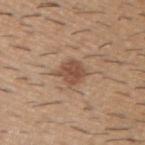This lesion was catalogued during total-body skin photography and was not selected for biopsy. Automated image analysis of the tile measured a lesion color around L≈51 a*≈19 b*≈30 in CIELAB and a normalized border contrast of about 7.5. The analysis additionally found an automated nevus-likeness rating near 90 out of 100 and lesion-presence confidence of about 100/100. The patient is a male roughly 60 years of age. About 3.5 mm across. A lesion tile, about 15 mm wide, cut from a 3D total-body photograph. This is a white-light tile. The lesion is located on the upper back.